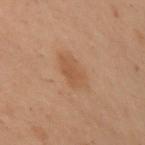Impression: Part of a total-body skin-imaging series; this lesion was reviewed on a skin check and was not flagged for biopsy. Clinical summary: On the left arm. The subject is a female in their mid- to late 50s. Longest diameter approximately 4.5 mm. A 15 mm close-up tile from a total-body photography series done for melanoma screening. The tile uses cross-polarized illumination.